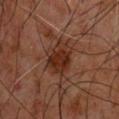This lesion was catalogued during total-body skin photography and was not selected for biopsy. This is a cross-polarized tile. A 15 mm crop from a total-body photograph taken for skin-cancer surveillance. The patient is a male aged 58 to 62. Automated image analysis of the tile measured a footprint of about 13 mm², a shape eccentricity near 0.75, and a symmetry-axis asymmetry near 0.25. It also reported internal color variation of about 4 on a 0–10 scale and radial color variation of about 1. From the upper back.